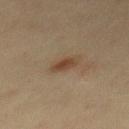follow-up — total-body-photography surveillance lesion; no biopsy | anatomic site — the back | size — ≈3 mm | illumination — cross-polarized | image source — ~15 mm tile from a whole-body skin photo | patient — male, aged approximately 40 | image-analysis metrics — a shape-asymmetry score of about 0.3 (0 = symmetric); a lesion color around L≈38 a*≈16 b*≈27 in CIELAB, about 8 CIELAB-L* units darker than the surrounding skin, and a lesion-to-skin contrast of about 8 (normalized; higher = more distinct); internal color variation of about 1.5 on a 0–10 scale and radial color variation of about 0.5; an automated nevus-likeness rating near 85 out of 100.